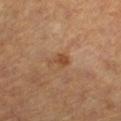workup = imaged on a skin check; not biopsied | anatomic site = the right lower leg | subject = female, roughly 60 years of age | imaging modality = 15 mm crop, total-body photography.